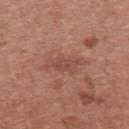The lesion was photographed on a routine skin check and not biopsied; there is no pathology result.
A female subject, about 40 years old.
Cropped from a whole-body photographic skin survey; the tile spans about 15 mm.
The lesion is located on the back.